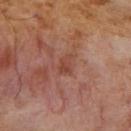  image:
    source: total-body photography crop
    field_of_view_mm: 15
  site: right upper arm
  automated_metrics:
    eccentricity: 0.85
    shape_asymmetry: 0.35
    cielab_L: 44
    cielab_a: 25
    cielab_b: 29
    vs_skin_darker_L: 7.0
    vs_skin_contrast_norm: 5.5
    border_irregularity_0_10: 4.0
    peripheral_color_asymmetry: 0.5
    nevus_likeness_0_100: 0
    lesion_detection_confidence_0_100: 100
  lesion_size:
    long_diameter_mm_approx: 2.5
  patient:
    sex: male
    age_approx: 55